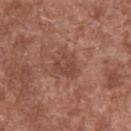No biopsy was performed on this lesion — it was imaged during a full skin examination and was not determined to be concerning. The tile uses white-light illumination. A male subject, about 45 years old. An algorithmic analysis of the crop reported an automated nevus-likeness rating near 0 out of 100 and lesion-presence confidence of about 100/100. A roughly 15 mm field-of-view crop from a total-body skin photograph. The lesion is on the upper back. Approximately 3 mm at its widest.